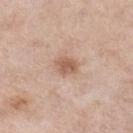Q: Is there a histopathology result?
A: total-body-photography surveillance lesion; no biopsy